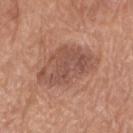Q: How was this image acquired?
A: total-body-photography crop, ~15 mm field of view
Q: Automated lesion metrics?
A: a border-irregularity index near 3/10, internal color variation of about 4.5 on a 0–10 scale, and radial color variation of about 1.5; a classifier nevus-likeness of about 5/100 and a detector confidence of about 100 out of 100 that the crop contains a lesion
Q: What is the lesion's diameter?
A: ≈7.5 mm
Q: Where on the body is the lesion?
A: the left upper arm
Q: How was the tile lit?
A: white-light illumination
Q: What are the patient's age and sex?
A: female, aged 73–77